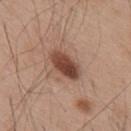Captured during whole-body skin photography for melanoma surveillance; the lesion was not biopsied.
The tile uses white-light illumination.
Cropped from a whole-body photographic skin survey; the tile spans about 15 mm.
The lesion is located on the back.
The lesion's longest dimension is about 4 mm.
A male patient, about 55 years old.